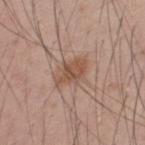The lesion was tiled from a total-body skin photograph and was not biopsied.
Measured at roughly 4 mm in maximum diameter.
Cropped from a total-body skin-imaging series; the visible field is about 15 mm.
On the upper back.
The patient is a male roughly 35 years of age.
The total-body-photography lesion software estimated a lesion area of about 7 mm², a shape eccentricity near 0.8, and a symmetry-axis asymmetry near 0.25. The analysis additionally found border irregularity of about 3 on a 0–10 scale, internal color variation of about 3 on a 0–10 scale, and peripheral color asymmetry of about 1.
Captured under white-light illumination.